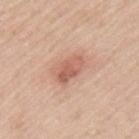The tile uses white-light illumination. Measured at roughly 4 mm in maximum diameter. Automated image analysis of the tile measured a lesion area of about 6.5 mm², an outline eccentricity of about 0.85 (0 = round, 1 = elongated), and two-axis asymmetry of about 0.2. And it measured a classifier nevus-likeness of about 30/100 and lesion-presence confidence of about 100/100. A male subject, aged around 45. The lesion is on the upper back. A roughly 15 mm field-of-view crop from a total-body skin photograph.